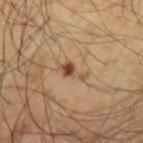Captured during whole-body skin photography for melanoma surveillance; the lesion was not biopsied.
A male subject aged 53–57.
This is a cross-polarized tile.
The lesion's longest dimension is about 3.5 mm.
An algorithmic analysis of the crop reported a border-irregularity rating of about 5.5/10, internal color variation of about 3 on a 0–10 scale, and peripheral color asymmetry of about 0.5. And it measured lesion-presence confidence of about 100/100.
Cropped from a whole-body photographic skin survey; the tile spans about 15 mm.
Located on the left upper arm.